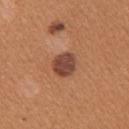This lesion was catalogued during total-body skin photography and was not selected for biopsy. Approximately 3 mm at its widest. On the right upper arm. The patient is a female aged 38–42. A 15 mm close-up extracted from a 3D total-body photography capture. Imaged with white-light lighting.